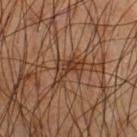| field | value |
|---|---|
| notes | catalogued during a skin exam; not biopsied |
| body site | the chest |
| patient | male, aged around 65 |
| automated lesion analysis | a mean CIELAB color near L≈36 a*≈19 b*≈29, roughly 9 lightness units darker than nearby skin, and a normalized lesion–skin contrast near 8; internal color variation of about 4.5 on a 0–10 scale and a peripheral color-asymmetry measure near 1.5 |
| image source | 15 mm crop, total-body photography |
| lighting | cross-polarized illumination |
| size | about 4 mm |